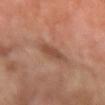Captured during whole-body skin photography for melanoma surveillance; the lesion was not biopsied.
Captured under cross-polarized illumination.
From the left forearm.
Cropped from a total-body skin-imaging series; the visible field is about 15 mm.
The lesion's longest dimension is about 3 mm.
A male patient aged approximately 65.